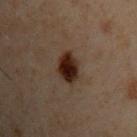Findings:
- workup — total-body-photography surveillance lesion; no biopsy
- automated lesion analysis — border irregularity of about 2 on a 0–10 scale and a within-lesion color-variation index near 5/10; an automated nevus-likeness rating near 100 out of 100
- body site — the upper back
- subject — male, aged 48 to 52
- lesion size — about 3.5 mm
- acquisition — ~15 mm crop, total-body skin-cancer survey
- illumination — cross-polarized illumination